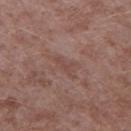Recorded during total-body skin imaging; not selected for excision or biopsy.
The subject is a male in their mid- to late 40s.
Captured under white-light illumination.
Measured at roughly 3 mm in maximum diameter.
Cropped from a total-body skin-imaging series; the visible field is about 15 mm.
The lesion is on the leg.